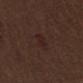Part of a total-body skin-imaging series; this lesion was reviewed on a skin check and was not flagged for biopsy.
The tile uses white-light illumination.
On the mid back.
This image is a 15 mm lesion crop taken from a total-body photograph.
A male patient, aged approximately 70.
Automated tile analysis of the lesion measured an average lesion color of about L≈22 a*≈17 b*≈17 (CIELAB), roughly 4 lightness units darker than nearby skin, and a lesion-to-skin contrast of about 5 (normalized; higher = more distinct). The software also gave internal color variation of about 2 on a 0–10 scale and radial color variation of about 0.5. The software also gave a detector confidence of about 100 out of 100 that the crop contains a lesion.
Measured at roughly 3 mm in maximum diameter.